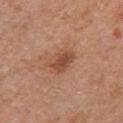The lesion was photographed on a routine skin check and not biopsied; there is no pathology result. Cropped from a whole-body photographic skin survey; the tile spans about 15 mm. A female subject, aged around 60. The lesion is on the right upper arm.